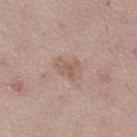No biopsy was performed on this lesion — it was imaged during a full skin examination and was not determined to be concerning.
Located on the leg.
Imaged with white-light lighting.
Automated tile analysis of the lesion measured an average lesion color of about L≈57 a*≈18 b*≈26 (CIELAB) and roughly 7 lightness units darker than nearby skin. It also reported a border-irregularity index near 4/10 and internal color variation of about 2.5 on a 0–10 scale. The analysis additionally found a classifier nevus-likeness of about 0/100.
A 15 mm close-up extracted from a 3D total-body photography capture.
A female patient, about 25 years old.
The recorded lesion diameter is about 3 mm.